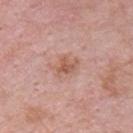notes=catalogued during a skin exam; not biopsied
anatomic site=the chest
tile lighting=white-light illumination
diameter=≈3 mm
image source=total-body-photography crop, ~15 mm field of view
patient=male, aged 53 to 57